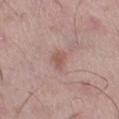notes=catalogued during a skin exam; not biopsied | image=total-body-photography crop, ~15 mm field of view | subject=male, aged approximately 55 | location=the right thigh.